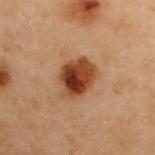Clinical impression: Captured during whole-body skin photography for melanoma surveillance; the lesion was not biopsied. Context: The lesion is located on the upper back. A female subject aged 58 to 62. Measured at roughly 4.5 mm in maximum diameter. Automated tile analysis of the lesion measured an area of roughly 12 mm², an eccentricity of roughly 0.55, and a symmetry-axis asymmetry near 0.15. And it measured an average lesion color of about L≈37 a*≈23 b*≈32 (CIELAB), roughly 15 lightness units darker than nearby skin, and a normalized border contrast of about 12.5. And it measured a border-irregularity index near 2/10, internal color variation of about 8.5 on a 0–10 scale, and radial color variation of about 3.5. The analysis additionally found a classifier nevus-likeness of about 100/100 and lesion-presence confidence of about 100/100. A roughly 15 mm field-of-view crop from a total-body skin photograph. This is a cross-polarized tile.